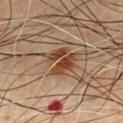Part of a total-body skin-imaging series; this lesion was reviewed on a skin check and was not flagged for biopsy. This image is a 15 mm lesion crop taken from a total-body photograph. The subject is a male roughly 60 years of age.